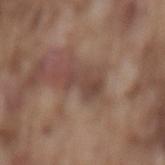Q: Was this lesion biopsied?
A: catalogued during a skin exam; not biopsied
Q: Lesion location?
A: the mid back
Q: What are the patient's age and sex?
A: male, aged approximately 75
Q: How was this image acquired?
A: 15 mm crop, total-body photography